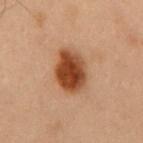workup = total-body-photography surveillance lesion; no biopsy
lighting = cross-polarized illumination
lesion diameter = about 5 mm
image = 15 mm crop, total-body photography
body site = the chest
patient = male, in their mid-50s